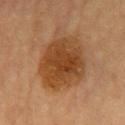No biopsy was performed on this lesion — it was imaged during a full skin examination and was not determined to be concerning. A female patient about 60 years old. Imaged with cross-polarized lighting. A roughly 15 mm field-of-view crop from a total-body skin photograph. From the chest.